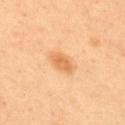Assessment:
The lesion was tiled from a total-body skin photograph and was not biopsied.
Context:
The patient is a female aged approximately 40. This is a cross-polarized tile. Approximately 3.5 mm at its widest. A close-up tile cropped from a whole-body skin photograph, about 15 mm across. Located on the upper back. The total-body-photography lesion software estimated a lesion color around L≈68 a*≈24 b*≈44 in CIELAB, roughly 10 lightness units darker than nearby skin, and a normalized lesion–skin contrast near 6.5. And it measured a border-irregularity index near 1/10, a within-lesion color-variation index near 2.5/10, and radial color variation of about 1.